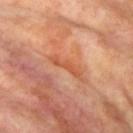The lesion's longest dimension is about 4 mm. Automated image analysis of the tile measured an average lesion color of about L≈56 a*≈30 b*≈39 (CIELAB), about 8 CIELAB-L* units darker than the surrounding skin, and a normalized lesion–skin contrast near 6.5. The software also gave a border-irregularity index near 6.5/10 and a within-lesion color-variation index near 0/10. The software also gave a classifier nevus-likeness of about 0/100 and lesion-presence confidence of about 70/100. The subject is a female aged approximately 75. The lesion is on the left upper arm. A lesion tile, about 15 mm wide, cut from a 3D total-body photograph.